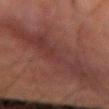Q: Was this lesion biopsied?
A: total-body-photography surveillance lesion; no biopsy
Q: Lesion location?
A: the right forearm
Q: What is the imaging modality?
A: total-body-photography crop, ~15 mm field of view
Q: Illumination type?
A: cross-polarized
Q: Who is the patient?
A: male, aged around 65